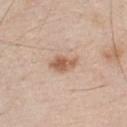| key | value |
|---|---|
| follow-up | no biopsy performed (imaged during a skin exam) |
| size | ≈3.5 mm |
| site | the chest |
| patient | male, aged approximately 45 |
| image source | 15 mm crop, total-body photography |